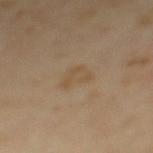Clinical impression:
The lesion was photographed on a routine skin check and not biopsied; there is no pathology result.
Background:
Imaged with cross-polarized lighting. The lesion-visualizer software estimated a lesion area of about 6 mm² and a symmetry-axis asymmetry near 0.35. And it measured a mean CIELAB color near L≈51 a*≈13 b*≈32, about 5 CIELAB-L* units darker than the surrounding skin, and a normalized border contrast of about 4.5. Cropped from a whole-body photographic skin survey; the tile spans about 15 mm. The subject is a male aged 63 to 67. On the mid back. Longest diameter approximately 3 mm.